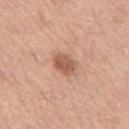This lesion was catalogued during total-body skin photography and was not selected for biopsy.
A 15 mm crop from a total-body photograph taken for skin-cancer surveillance.
A female subject aged 63–67.
This is a white-light tile.
Longest diameter approximately 3 mm.
Located on the leg.